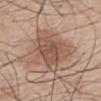Assessment: Recorded during total-body skin imaging; not selected for excision or biopsy. Image and clinical context: A 15 mm crop from a total-body photograph taken for skin-cancer surveillance. A male subject, about 75 years old. This is a white-light tile. On the back. Automated tile analysis of the lesion measured a nevus-likeness score of about 5/100.